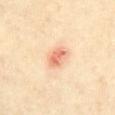This lesion was catalogued during total-body skin photography and was not selected for biopsy. A region of skin cropped from a whole-body photographic capture, roughly 15 mm wide. Automated image analysis of the tile measured an area of roughly 4 mm², an outline eccentricity of about 0.8 (0 = round, 1 = elongated), and two-axis asymmetry of about 0.2. The analysis additionally found a lesion–skin lightness drop of about 13 and a lesion-to-skin contrast of about 7.5 (normalized; higher = more distinct). And it measured a border-irregularity rating of about 2/10, a within-lesion color-variation index near 3/10, and radial color variation of about 1. The analysis additionally found a lesion-detection confidence of about 100/100. The lesion is on the front of the torso. A female patient aged around 70. Measured at roughly 3 mm in maximum diameter.